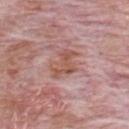follow-up — imaged on a skin check; not biopsied
patient — male, aged 78 to 82
anatomic site — the chest
acquisition — ~15 mm crop, total-body skin-cancer survey
image-analysis metrics — an eccentricity of roughly 0.75 and a shape-asymmetry score of about 0.45 (0 = symmetric); an automated nevus-likeness rating near 0 out of 100 and a detector confidence of about 100 out of 100 that the crop contains a lesion
illumination — white-light